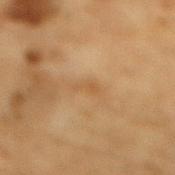Notes:
* follow-up — total-body-photography surveillance lesion; no biopsy
* body site — the mid back
* patient — male, roughly 85 years of age or older
* illumination — cross-polarized
* image — 15 mm crop, total-body photography